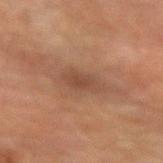This lesion was catalogued during total-body skin photography and was not selected for biopsy. From the arm. The lesion's longest dimension is about 4.5 mm. A male patient, aged 73 to 77. A roughly 15 mm field-of-view crop from a total-body skin photograph.